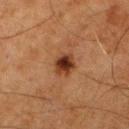Recorded during total-body skin imaging; not selected for excision or biopsy.
Cropped from a total-body skin-imaging series; the visible field is about 15 mm.
From the leg.
A male patient about 80 years old.
About 3 mm across.